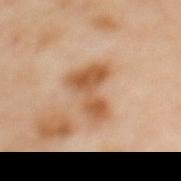Q: Was a biopsy performed?
A: total-body-photography surveillance lesion; no biopsy